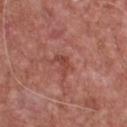notes = no biopsy performed (imaged during a skin exam)
acquisition = 15 mm crop, total-body photography
automated metrics = a mean CIELAB color near L≈45 a*≈28 b*≈27, a lesion–skin lightness drop of about 8, and a normalized lesion–skin contrast near 6; an automated nevus-likeness rating near 0 out of 100 and lesion-presence confidence of about 100/100
patient = male, aged 63 to 67
lighting = white-light
lesion size = about 3 mm
body site = the chest